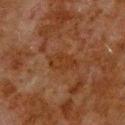| field | value |
|---|---|
| tile lighting | cross-polarized illumination |
| image | ~15 mm tile from a whole-body skin photo |
| patient | male, aged 78–82 |
| anatomic site | the upper back |